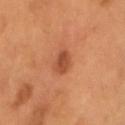notes: imaged on a skin check; not biopsied
subject: male, approximately 55 years of age
lesion size: ≈2.5 mm
imaging modality: 15 mm crop, total-body photography
anatomic site: the head or neck
automated metrics: a footprint of about 4 mm², an eccentricity of roughly 0.65, and a symmetry-axis asymmetry near 0.25; an average lesion color of about L≈44 a*≈26 b*≈34 (CIELAB); peripheral color asymmetry of about 1; a nevus-likeness score of about 80/100 and a lesion-detection confidence of about 100/100
illumination: cross-polarized illumination